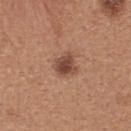Assessment:
Recorded during total-body skin imaging; not selected for excision or biopsy.
Background:
A lesion tile, about 15 mm wide, cut from a 3D total-body photograph. Located on the upper back. A female patient, aged 38 to 42. Captured under white-light illumination. Measured at roughly 2.5 mm in maximum diameter.